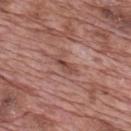{"biopsy_status": "not biopsied; imaged during a skin examination"}